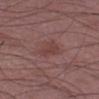Q: What is the lesion's diameter?
A: ≈3 mm
Q: Where on the body is the lesion?
A: the left forearm
Q: What is the imaging modality?
A: total-body-photography crop, ~15 mm field of view
Q: Illumination type?
A: white-light
Q: What are the patient's age and sex?
A: male, aged approximately 50